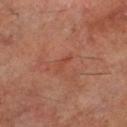  biopsy_status: not biopsied; imaged during a skin examination
  patient:
    sex: male
    age_approx: 60
  lighting: cross-polarized
  lesion_size:
    long_diameter_mm_approx: 2.5
  image:
    source: total-body photography crop
    field_of_view_mm: 15
  site: left lower leg